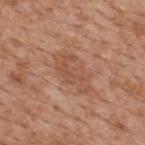Clinical impression:
The lesion was tiled from a total-body skin photograph and was not biopsied.
Clinical summary:
Captured under white-light illumination. On the upper back. A male subject, approximately 65 years of age. A 15 mm close-up extracted from a 3D total-body photography capture.